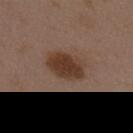follow-up = catalogued during a skin exam; not biopsied
patient = female, about 35 years old
anatomic site = the upper back
tile lighting = white-light illumination
imaging modality = ~15 mm tile from a whole-body skin photo
lesion size = about 5 mm